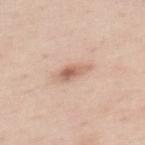Recorded during total-body skin imaging; not selected for excision or biopsy.
From the upper back.
Measured at roughly 3.5 mm in maximum diameter.
Automated tile analysis of the lesion measured border irregularity of about 3 on a 0–10 scale. The analysis additionally found a lesion-detection confidence of about 100/100.
A roughly 15 mm field-of-view crop from a total-body skin photograph.
A female patient, aged around 35.
Imaged with white-light lighting.